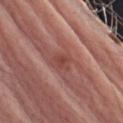Case summary:
- notes: no biopsy performed (imaged during a skin exam)
- image source: ~15 mm tile from a whole-body skin photo
- body site: the left upper arm
- subject: male, approximately 65 years of age